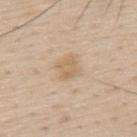This lesion was catalogued during total-body skin photography and was not selected for biopsy.
The patient is a male in their mid- to late 70s.
A region of skin cropped from a whole-body photographic capture, roughly 15 mm wide.
The total-body-photography lesion software estimated a mean CIELAB color near L≈64 a*≈14 b*≈33 and a lesion-to-skin contrast of about 5.5 (normalized; higher = more distinct). It also reported border irregularity of about 2 on a 0–10 scale, a color-variation rating of about 1.5/10, and radial color variation of about 0.5.
This is a white-light tile.
On the upper back.
Measured at roughly 2.5 mm in maximum diameter.